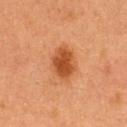Q: Lesion size?
A: ≈4.5 mm
Q: What are the patient's age and sex?
A: female, aged 38–42
Q: Where on the body is the lesion?
A: the upper back
Q: Illumination type?
A: cross-polarized illumination
Q: Automated lesion metrics?
A: a lesion color around L≈44 a*≈26 b*≈38 in CIELAB, about 12 CIELAB-L* units darker than the surrounding skin, and a lesion-to-skin contrast of about 9.5 (normalized; higher = more distinct); an automated nevus-likeness rating near 100 out of 100 and a lesion-detection confidence of about 100/100
Q: What is the imaging modality?
A: ~15 mm crop, total-body skin-cancer survey